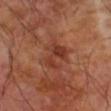- patient: male, aged 68–72
- tile lighting: cross-polarized
- image source: ~15 mm tile from a whole-body skin photo
- automated lesion analysis: roughly 8 lightness units darker than nearby skin and a normalized lesion–skin contrast near 6.5; a classifier nevus-likeness of about 5/100 and a detector confidence of about 100 out of 100 that the crop contains a lesion
- diameter: about 3.5 mm
- site: the right upper arm